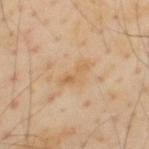The lesion was photographed on a routine skin check and not biopsied; there is no pathology result. Automated image analysis of the tile measured an area of roughly 5 mm², an eccentricity of roughly 0.9, and a shape-asymmetry score of about 0.35 (0 = symmetric). The analysis additionally found a mean CIELAB color near L≈63 a*≈17 b*≈38, a lesion–skin lightness drop of about 6, and a normalized lesion–skin contrast near 5.5. And it measured a border-irregularity rating of about 4.5/10, internal color variation of about 3.5 on a 0–10 scale, and a peripheral color-asymmetry measure near 1. A male patient, in their mid- to late 50s. The tile uses cross-polarized illumination. Longest diameter approximately 4 mm. This image is a 15 mm lesion crop taken from a total-body photograph. Located on the upper back.